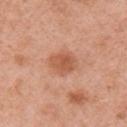| key | value |
|---|---|
| workup | no biopsy performed (imaged during a skin exam) |
| subject | female, aged 48–52 |
| location | the right upper arm |
| image | total-body-photography crop, ~15 mm field of view |